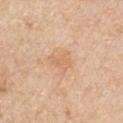workup: total-body-photography surveillance lesion; no biopsy | image: ~15 mm crop, total-body skin-cancer survey | automated lesion analysis: a lesion–skin lightness drop of about 6 and a lesion-to-skin contrast of about 4.5 (normalized; higher = more distinct); a detector confidence of about 100 out of 100 that the crop contains a lesion | patient: male, approximately 60 years of age | anatomic site: the front of the torso | tile lighting: white-light | diameter: ~3 mm (longest diameter).